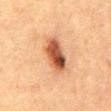Assessment:
No biopsy was performed on this lesion — it was imaged during a full skin examination and was not determined to be concerning.
Background:
The lesion is located on the abdomen. A female patient about 60 years old. A lesion tile, about 15 mm wide, cut from a 3D total-body photograph. Longest diameter approximately 5 mm.